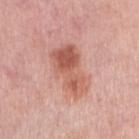Impression:
The lesion was tiled from a total-body skin photograph and was not biopsied.
Clinical summary:
The lesion-visualizer software estimated an area of roughly 17 mm², an outline eccentricity of about 0.85 (0 = round, 1 = elongated), and two-axis asymmetry of about 0.2. It also reported a mean CIELAB color near L≈59 a*≈26 b*≈29, about 11 CIELAB-L* units darker than the surrounding skin, and a normalized lesion–skin contrast near 7.5. It also reported a within-lesion color-variation index near 7.5/10 and a peripheral color-asymmetry measure near 3. It also reported a classifier nevus-likeness of about 50/100 and a lesion-detection confidence of about 100/100. A close-up tile cropped from a whole-body skin photograph, about 15 mm across. A female patient, aged approximately 65. Located on the right upper arm. The tile uses white-light illumination.